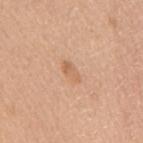biopsy status — imaged on a skin check; not biopsied
site — the mid back
patient — female, about 70 years old
image — ~15 mm crop, total-body skin-cancer survey